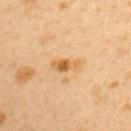Notes:
• workup: no biopsy performed (imaged during a skin exam)
• anatomic site: the arm
• image: ~15 mm tile from a whole-body skin photo
• diameter: ≈3.5 mm
• subject: female, approximately 40 years of age
• tile lighting: cross-polarized illumination